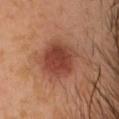Impression: Imaged during a routine full-body skin examination; the lesion was not biopsied and no histopathology is available. Clinical summary: The recorded lesion diameter is about 4.5 mm. The total-body-photography lesion software estimated a footprint of about 15 mm², an eccentricity of roughly 0.35, and a symmetry-axis asymmetry near 0.1. And it measured a lesion–skin lightness drop of about 11 and a normalized border contrast of about 8.5. It also reported a border-irregularity rating of about 1.5/10. The software also gave a classifier nevus-likeness of about 95/100 and a lesion-detection confidence of about 100/100. A close-up tile cropped from a whole-body skin photograph, about 15 mm across. Captured under cross-polarized illumination. A male subject, approximately 30 years of age. From the head or neck.